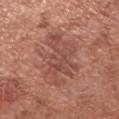Findings:
• biopsy status: total-body-photography surveillance lesion; no biopsy
• patient: female, in their 50s
• lesion size: ~7.5 mm (longest diameter)
• image source: ~15 mm crop, total-body skin-cancer survey
• lighting: white-light illumination
• body site: the left forearm